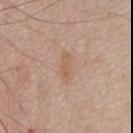The lesion was tiled from a total-body skin photograph and was not biopsied. About 3.5 mm across. This image is a 15 mm lesion crop taken from a total-body photograph. A male subject, approximately 70 years of age. The lesion is located on the chest. Captured under white-light illumination.